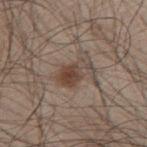Q: Is there a histopathology result?
A: total-body-photography surveillance lesion; no biopsy
Q: Where on the body is the lesion?
A: the upper back
Q: What kind of image is this?
A: 15 mm crop, total-body photography
Q: How large is the lesion?
A: ≈4 mm
Q: Patient demographics?
A: male, in their mid-40s